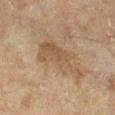workup=catalogued during a skin exam; not biopsied | subject=female, roughly 60 years of age | image=~15 mm crop, total-body skin-cancer survey | location=the right lower leg | lighting=cross-polarized.